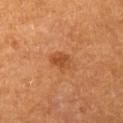Q: Is there a histopathology result?
A: no biopsy performed (imaged during a skin exam)
Q: Illumination type?
A: cross-polarized
Q: How was this image acquired?
A: total-body-photography crop, ~15 mm field of view
Q: Where on the body is the lesion?
A: the left upper arm
Q: How large is the lesion?
A: ~2.5 mm (longest diameter)
Q: Who is the patient?
A: female, aged around 65